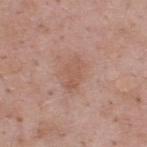{"biopsy_status": "not biopsied; imaged during a skin examination", "site": "upper back", "automated_metrics": {"border_irregularity_0_10": 4.0, "color_variation_0_10": 2.0, "peripheral_color_asymmetry": 0.5, "lesion_detection_confidence_0_100": 100}, "lesion_size": {"long_diameter_mm_approx": 3.5}, "image": {"source": "total-body photography crop", "field_of_view_mm": 15}, "lighting": "white-light", "patient": {"sex": "male", "age_approx": 55}}